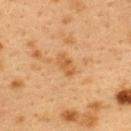imaging modality=total-body-photography crop, ~15 mm field of view | patient=female, roughly 40 years of age | lesion diameter=about 3 mm | site=the upper back.